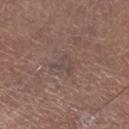Assessment: Recorded during total-body skin imaging; not selected for excision or biopsy. Clinical summary: This is a white-light tile. A male patient, aged approximately 65. A close-up tile cropped from a whole-body skin photograph, about 15 mm across. The lesion's longest dimension is about 3 mm. From the left lower leg. Automated tile analysis of the lesion measured a lesion area of about 4 mm², an outline eccentricity of about 0.8 (0 = round, 1 = elongated), and two-axis asymmetry of about 0.65. It also reported a mean CIELAB color near L≈44 a*≈14 b*≈19 and about 5 CIELAB-L* units darker than the surrounding skin. The analysis additionally found border irregularity of about 6.5 on a 0–10 scale and a color-variation rating of about 1/10.